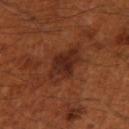Q: Is there a histopathology result?
A: total-body-photography surveillance lesion; no biopsy
Q: What is the lesion's diameter?
A: ≈5.5 mm
Q: Automated lesion metrics?
A: an area of roughly 11 mm² and a symmetry-axis asymmetry near 0.25; a mean CIELAB color near L≈27 a*≈23 b*≈28 and a lesion–skin lightness drop of about 8; a border-irregularity index near 3/10, a color-variation rating of about 3.5/10, and radial color variation of about 1
Q: How was this image acquired?
A: ~15 mm tile from a whole-body skin photo
Q: What lighting was used for the tile?
A: cross-polarized
Q: What is the anatomic site?
A: the arm
Q: What are the patient's age and sex?
A: male, aged 48 to 52